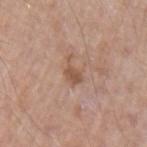biopsy status — catalogued during a skin exam; not biopsied